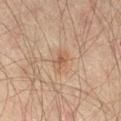subject = male, aged around 45; anatomic site = the right thigh; image source = ~15 mm tile from a whole-body skin photo.